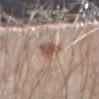{"biopsy_status": "not biopsied; imaged during a skin examination", "site": "arm", "image": {"source": "total-body photography crop", "field_of_view_mm": 15}, "patient": {"sex": "male", "age_approx": 80}}